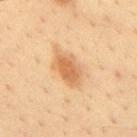notes: no biopsy performed (imaged during a skin exam) | imaging modality: total-body-photography crop, ~15 mm field of view | body site: the mid back | patient: male, roughly 35 years of age.